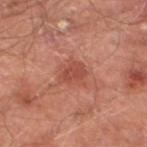Assessment: Imaged during a routine full-body skin examination; the lesion was not biopsied and no histopathology is available. Background: The tile uses cross-polarized illumination. Measured at roughly 3 mm in maximum diameter. A male patient about 65 years old. A 15 mm close-up tile from a total-body photography series done for melanoma screening. The lesion is on the left lower leg.